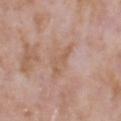Part of a total-body skin-imaging series; this lesion was reviewed on a skin check and was not flagged for biopsy. Automated tile analysis of the lesion measured a lesion area of about 5 mm², a shape eccentricity near 0.95, and two-axis asymmetry of about 0.55. A 15 mm crop from a total-body photograph taken for skin-cancer surveillance. The lesion is located on the head or neck. This is a white-light tile. A male patient, roughly 70 years of age.